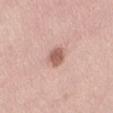The lesion was tiled from a total-body skin photograph and was not biopsied.
A female patient, about 50 years old.
A 15 mm close-up tile from a total-body photography series done for melanoma screening.
Imaged with white-light lighting.
Approximately 3 mm at its widest.
From the right thigh.